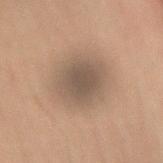Imaged during a routine full-body skin examination; the lesion was not biopsied and no histopathology is available. The patient is a male approximately 55 years of age. Longest diameter approximately 5.5 mm. Automated image analysis of the tile measured an outline eccentricity of about 0.55 (0 = round, 1 = elongated) and a shape-asymmetry score of about 0.15 (0 = symmetric). The software also gave border irregularity of about 1.5 on a 0–10 scale and peripheral color asymmetry of about 0.5. The analysis additionally found a nevus-likeness score of about 20/100 and a detector confidence of about 95 out of 100 that the crop contains a lesion. On the left arm. The tile uses cross-polarized illumination. A 15 mm close-up extracted from a 3D total-body photography capture.